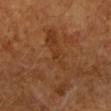biopsy status: imaged on a skin check; not biopsied
subject: aged around 70
site: the arm
image source: ~15 mm crop, total-body skin-cancer survey